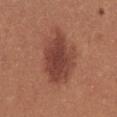Q: Was this lesion biopsied?
A: no biopsy performed (imaged during a skin exam)
Q: Who is the patient?
A: female, roughly 25 years of age
Q: What is the imaging modality?
A: 15 mm crop, total-body photography
Q: Lesion location?
A: the chest
Q: How large is the lesion?
A: about 7 mm
Q: Illumination type?
A: white-light